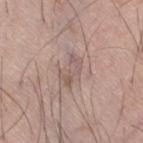| feature | finding |
|---|---|
| follow-up | imaged on a skin check; not biopsied |